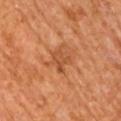| field | value |
|---|---|
| follow-up | catalogued during a skin exam; not biopsied |
| image source | 15 mm crop, total-body photography |
| tile lighting | cross-polarized illumination |
| diameter | about 3 mm |
| subject | male, approximately 65 years of age |
| body site | the right upper arm |